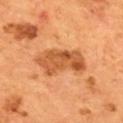notes: no biopsy performed (imaged during a skin exam); location: the mid back; illumination: cross-polarized illumination; subject: male, about 55 years old; lesion diameter: ≈6.5 mm; image: total-body-photography crop, ~15 mm field of view.